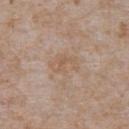<tbp_lesion>
<biopsy_status>not biopsied; imaged during a skin examination</biopsy_status>
<site>abdomen</site>
<lighting>white-light</lighting>
<patient>
  <sex>male</sex>
  <age_approx>65</age_approx>
</patient>
<image>
  <source>total-body photography crop</source>
  <field_of_view_mm>15</field_of_view_mm>
</image>
</tbp_lesion>